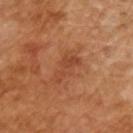Imaged during a routine full-body skin examination; the lesion was not biopsied and no histopathology is available.
A male subject, in their mid- to late 60s.
The lesion's longest dimension is about 4.5 mm.
A 15 mm close-up tile from a total-body photography series done for melanoma screening.
Imaged with cross-polarized lighting.